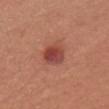Notes:
• biopsy status · catalogued during a skin exam; not biopsied
• subject · female, aged approximately 35
• site · the upper back
• lesion diameter · ≈3.5 mm
• acquisition · 15 mm crop, total-body photography
• tile lighting · white-light illumination